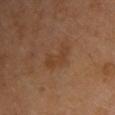Recorded during total-body skin imaging; not selected for excision or biopsy. The lesion-visualizer software estimated an outline eccentricity of about 0.8 (0 = round, 1 = elongated) and two-axis asymmetry of about 0.45. It also reported a classifier nevus-likeness of about 0/100. The patient is a male roughly 70 years of age. The lesion's longest dimension is about 3.5 mm. Imaged with cross-polarized lighting. The lesion is located on the upper back. Cropped from a whole-body photographic skin survey; the tile spans about 15 mm.